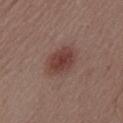  biopsy_status: not biopsied; imaged during a skin examination
  lesion_size:
    long_diameter_mm_approx: 4.5
  image:
    source: total-body photography crop
    field_of_view_mm: 15
  site: abdomen
  automated_metrics:
    area_mm2_approx: 12.0
    eccentricity: 0.7
    shape_asymmetry: 0.15
    cielab_L: 41
    cielab_a: 21
    cielab_b: 22
    vs_skin_darker_L: 9.0
    border_irregularity_0_10: 1.5
    color_variation_0_10: 3.5
    peripheral_color_asymmetry: 1.0
  lighting: white-light
  patient:
    sex: male
    age_approx: 55